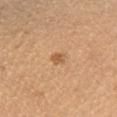Captured during whole-body skin photography for melanoma surveillance; the lesion was not biopsied. The lesion is on the right lower leg. A 15 mm crop from a total-body photograph taken for skin-cancer surveillance. A male patient aged 48–52.